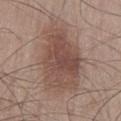notes: catalogued during a skin exam; not biopsied
patient: male, in their mid- to late 50s
body site: the left thigh
tile lighting: white-light
imaging modality: ~15 mm tile from a whole-body skin photo